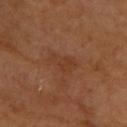Impression:
The lesion was tiled from a total-body skin photograph and was not biopsied.
Background:
A region of skin cropped from a whole-body photographic capture, roughly 15 mm wide. The patient is a male aged around 65. The recorded lesion diameter is about 4 mm. From the upper back. Captured under cross-polarized illumination. Automated image analysis of the tile measured a footprint of about 6.5 mm², a shape eccentricity near 0.85, and a shape-asymmetry score of about 0.5 (0 = symmetric). The software also gave a border-irregularity rating of about 5/10, a color-variation rating of about 2/10, and a peripheral color-asymmetry measure near 1.